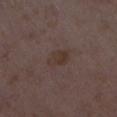Assessment: No biopsy was performed on this lesion — it was imaged during a full skin examination and was not determined to be concerning. Clinical summary: A 15 mm close-up extracted from a 3D total-body photography capture. A female subject, about 35 years old. The lesion is located on the leg. The lesion's longest dimension is about 3 mm.